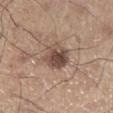- notes: total-body-photography surveillance lesion; no biopsy
- lesion diameter: about 3.5 mm
- site: the left lower leg
- patient: male, aged 53 to 57
- lighting: white-light illumination
- image source: ~15 mm tile from a whole-body skin photo